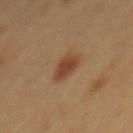image source: total-body-photography crop, ~15 mm field of view | subject: male, aged 48–52 | lesion diameter: about 3.5 mm | body site: the mid back.